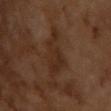Acquisition and patient details: The patient is a male in their mid- to late 60s. A close-up tile cropped from a whole-body skin photograph, about 15 mm across. Measured at roughly 5 mm in maximum diameter. Automated image analysis of the tile measured a lesion color around L≈26 a*≈17 b*≈25 in CIELAB, a lesion–skin lightness drop of about 5, and a normalized lesion–skin contrast near 6. And it measured a classifier nevus-likeness of about 0/100 and a detector confidence of about 100 out of 100 that the crop contains a lesion. Captured under cross-polarized illumination.